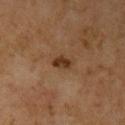Captured during whole-body skin photography for melanoma surveillance; the lesion was not biopsied.
About 2.5 mm across.
This is a cross-polarized tile.
From the left upper arm.
A roughly 15 mm field-of-view crop from a total-body skin photograph.
A female subject, approximately 60 years of age.
An algorithmic analysis of the crop reported a symmetry-axis asymmetry near 0.35. And it measured an average lesion color of about L≈30 a*≈17 b*≈28 (CIELAB), about 10 CIELAB-L* units darker than the surrounding skin, and a lesion-to-skin contrast of about 9.5 (normalized; higher = more distinct). The analysis additionally found a within-lesion color-variation index near 2.5/10 and radial color variation of about 1.